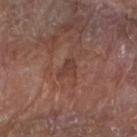Recorded during total-body skin imaging; not selected for excision or biopsy. About 2.5 mm across. The lesion is on the right forearm. The patient is a male aged approximately 85 or older. The total-body-photography lesion software estimated a lesion area of about 3.5 mm². The software also gave a mean CIELAB color near L≈39 a*≈21 b*≈24, a lesion–skin lightness drop of about 6, and a normalized lesion–skin contrast near 5.5. And it measured an automated nevus-likeness rating near 0 out of 100 and lesion-presence confidence of about 100/100. A 15 mm close-up extracted from a 3D total-body photography capture.